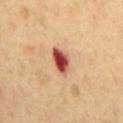notes: catalogued during a skin exam; not biopsied
illumination: cross-polarized illumination
lesion diameter: ≈4 mm
image: ~15 mm crop, total-body skin-cancer survey
patient: male, aged approximately 50
body site: the chest